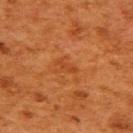Assessment:
Imaged during a routine full-body skin examination; the lesion was not biopsied and no histopathology is available.
Image and clinical context:
The lesion is on the back. The subject is a female roughly 50 years of age. The total-body-photography lesion software estimated an area of roughly 2 mm², a shape eccentricity near 0.95, and two-axis asymmetry of about 0.45. The software also gave a lesion color around L≈37 a*≈26 b*≈36 in CIELAB and a lesion-to-skin contrast of about 5 (normalized; higher = more distinct). The lesion's longest dimension is about 2.5 mm. A region of skin cropped from a whole-body photographic capture, roughly 15 mm wide.